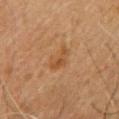Part of a total-body skin-imaging series; this lesion was reviewed on a skin check and was not flagged for biopsy.
A male patient, in their mid- to late 60s.
Imaged with cross-polarized lighting.
Longest diameter approximately 3.5 mm.
A roughly 15 mm field-of-view crop from a total-body skin photograph.
Located on the chest.
Automated tile analysis of the lesion measured an area of roughly 4.5 mm², an outline eccentricity of about 0.85 (0 = round, 1 = elongated), and a symmetry-axis asymmetry near 0.4. It also reported a lesion color around L≈41 a*≈19 b*≈33 in CIELAB, roughly 6 lightness units darker than nearby skin, and a normalized lesion–skin contrast near 6. The software also gave a border-irregularity index near 4.5/10, a within-lesion color-variation index near 2/10, and peripheral color asymmetry of about 0.5. And it measured a classifier nevus-likeness of about 5/100 and lesion-presence confidence of about 100/100.